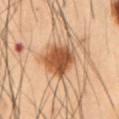notes = catalogued during a skin exam; not biopsied
location = the abdomen
patient = male, approximately 55 years of age
image = ~15 mm crop, total-body skin-cancer survey